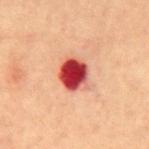| feature | finding |
|---|---|
| notes | no biopsy performed (imaged during a skin exam) |
| subject | male, aged approximately 70 |
| site | the mid back |
| lesion size | ≈3.5 mm |
| tile lighting | cross-polarized |
| imaging modality | ~15 mm tile from a whole-body skin photo |